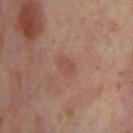Imaged during a routine full-body skin examination; the lesion was not biopsied and no histopathology is available. On the left thigh. A 15 mm close-up tile from a total-body photography series done for melanoma screening. Automated image analysis of the tile measured a lesion color around L≈50 a*≈22 b*≈28 in CIELAB, about 5 CIELAB-L* units darker than the surrounding skin, and a normalized border contrast of about 5. The analysis additionally found an automated nevus-likeness rating near 30 out of 100 and lesion-presence confidence of about 100/100. The subject is a female aged approximately 55. Imaged with cross-polarized lighting. Longest diameter approximately 2.5 mm.